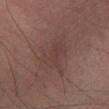No biopsy was performed on this lesion — it was imaged during a full skin examination and was not determined to be concerning.
Captured under cross-polarized illumination.
On the left lower leg.
The lesion's longest dimension is about 6 mm.
A male subject in their mid-50s.
Cropped from a whole-body photographic skin survey; the tile spans about 15 mm.